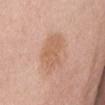Q: Is there a histopathology result?
A: no biopsy performed (imaged during a skin exam)
Q: What is the anatomic site?
A: the abdomen
Q: Automated lesion metrics?
A: a lesion color around L≈61 a*≈20 b*≈32 in CIELAB and a normalized border contrast of about 6; an automated nevus-likeness rating near 0 out of 100 and a lesion-detection confidence of about 100/100
Q: How was the tile lit?
A: white-light
Q: What kind of image is this?
A: 15 mm crop, total-body photography
Q: Patient demographics?
A: female, aged 63 to 67